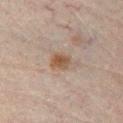notes: imaged on a skin check; not biopsied
body site: the right lower leg
acquisition: ~15 mm crop, total-body skin-cancer survey
patient: male, approximately 75 years of age
diameter: ≈3 mm
automated lesion analysis: border irregularity of about 2 on a 0–10 scale and peripheral color asymmetry of about 1.5; a classifier nevus-likeness of about 85/100 and a lesion-detection confidence of about 100/100
lighting: cross-polarized illumination